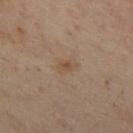{
  "biopsy_status": "not biopsied; imaged during a skin examination",
  "site": "chest",
  "image": {
    "source": "total-body photography crop",
    "field_of_view_mm": 15
  },
  "automated_metrics": {
    "peripheral_color_asymmetry": 1.0,
    "nevus_likeness_0_100": 0,
    "lesion_detection_confidence_0_100": 100
  },
  "patient": {
    "sex": "female",
    "age_approx": 55
  },
  "lighting": "cross-polarized"
}